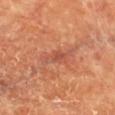Findings:
• workup — no biopsy performed (imaged during a skin exam)
• image-analysis metrics — a lesion area of about 5 mm², an outline eccentricity of about 0.9 (0 = round, 1 = elongated), and two-axis asymmetry of about 0.4; a lesion-to-skin contrast of about 5.5 (normalized; higher = more distinct); a peripheral color-asymmetry measure near 1; a classifier nevus-likeness of about 0/100 and a lesion-detection confidence of about 95/100
• imaging modality — total-body-photography crop, ~15 mm field of view
• patient — female, in their 80s
• location — the chest
• tile lighting — cross-polarized
• lesion size — ≈4 mm